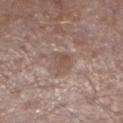notes: imaged on a skin check; not biopsied
patient: male, approximately 45 years of age
body site: the leg
tile lighting: white-light illumination
diameter: ~2.5 mm (longest diameter)
image source: ~15 mm crop, total-body skin-cancer survey